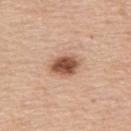{
  "biopsy_status": "not biopsied; imaged during a skin examination",
  "lesion_size": {
    "long_diameter_mm_approx": 3.5
  },
  "image": {
    "source": "total-body photography crop",
    "field_of_view_mm": 15
  },
  "lighting": "white-light",
  "automated_metrics": {
    "border_irregularity_0_10": 2.0,
    "color_variation_0_10": 4.5,
    "peripheral_color_asymmetry": 1.5
  },
  "patient": {
    "sex": "male",
    "age_approx": 80
  },
  "site": "upper back"
}